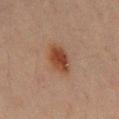Clinical impression: This lesion was catalogued during total-body skin photography and was not selected for biopsy. Image and clinical context: Located on the mid back. A male patient, in their mid- to late 40s. This image is a 15 mm lesion crop taken from a total-body photograph.